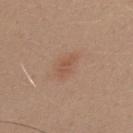No biopsy was performed on this lesion — it was imaged during a full skin examination and was not determined to be concerning. Located on the upper back. This image is a 15 mm lesion crop taken from a total-body photograph. A male patient aged approximately 30.